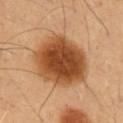Clinical impression:
Captured during whole-body skin photography for melanoma surveillance; the lesion was not biopsied.
Acquisition and patient details:
The lesion is on the chest. A lesion tile, about 15 mm wide, cut from a 3D total-body photograph. Measured at roughly 7.5 mm in maximum diameter. A male subject, in their mid- to late 50s.